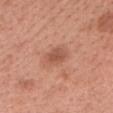Assessment: Part of a total-body skin-imaging series; this lesion was reviewed on a skin check and was not flagged for biopsy. Clinical summary: This is a white-light tile. From the head or neck. The lesion's longest dimension is about 3 mm. A 15 mm crop from a total-body photograph taken for skin-cancer surveillance. A female subject, aged 48 to 52.